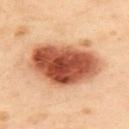| key | value |
|---|---|
| tile lighting | cross-polarized illumination |
| patient | female, aged around 40 |
| body site | the upper back |
| image source | ~15 mm tile from a whole-body skin photo |
| automated lesion analysis | a border-irregularity index near 2/10, a within-lesion color-variation index near 10/10, and peripheral color asymmetry of about 4 |